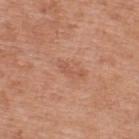No biopsy was performed on this lesion — it was imaged during a full skin examination and was not determined to be concerning. The recorded lesion diameter is about 3.5 mm. A male subject, aged approximately 70. On the upper back. A lesion tile, about 15 mm wide, cut from a 3D total-body photograph.